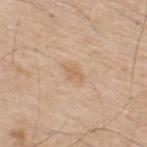notes = imaged on a skin check; not biopsied
location = the upper back
lighting = white-light illumination
subject = male, approximately 70 years of age
acquisition = ~15 mm tile from a whole-body skin photo